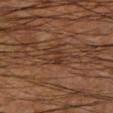This lesion was catalogued during total-body skin photography and was not selected for biopsy. An algorithmic analysis of the crop reported an average lesion color of about L≈33 a*≈18 b*≈28 (CIELAB), about 6 CIELAB-L* units darker than the surrounding skin, and a normalized lesion–skin contrast near 6. A lesion tile, about 15 mm wide, cut from a 3D total-body photograph. The recorded lesion diameter is about 3 mm. The tile uses cross-polarized illumination. From the left lower leg. The subject is a male aged 58 to 62.